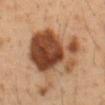Recorded during total-body skin imaging; not selected for excision or biopsy. About 7.5 mm across. The tile uses cross-polarized illumination. The total-body-photography lesion software estimated an average lesion color of about L≈46 a*≈22 b*≈33 (CIELAB), about 18 CIELAB-L* units darker than the surrounding skin, and a normalized border contrast of about 12.5. It also reported a border-irregularity index near 4/10, internal color variation of about 9.5 on a 0–10 scale, and radial color variation of about 3.5. It also reported a nevus-likeness score of about 30/100 and a lesion-detection confidence of about 100/100. A male subject aged 48–52. Located on the abdomen. A lesion tile, about 15 mm wide, cut from a 3D total-body photograph.